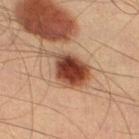– biopsy status · catalogued during a skin exam; not biopsied
– body site · the left thigh
– patient · male, aged approximately 40
– tile lighting · cross-polarized illumination
– image source · 15 mm crop, total-body photography